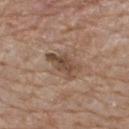follow-up: total-body-photography surveillance lesion; no biopsy
automated lesion analysis: an area of roughly 7.5 mm²; a mean CIELAB color near L≈47 a*≈16 b*≈28, about 10 CIELAB-L* units darker than the surrounding skin, and a normalized border contrast of about 8; a nevus-likeness score of about 0/100
lesion size: ≈4 mm
site: the upper back
image source: total-body-photography crop, ~15 mm field of view
patient: male, aged approximately 80